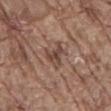| feature | finding |
|---|---|
| workup | no biopsy performed (imaged during a skin exam) |
| automated lesion analysis | border irregularity of about 6 on a 0–10 scale, internal color variation of about 3 on a 0–10 scale, and a peripheral color-asymmetry measure near 0.5; an automated nevus-likeness rating near 10 out of 100 and a detector confidence of about 75 out of 100 that the crop contains a lesion |
| patient | male, approximately 80 years of age |
| illumination | white-light |
| size | ≈3.5 mm |
| image | 15 mm crop, total-body photography |
| location | the front of the torso |